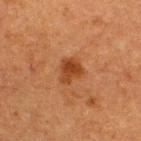The lesion was tiled from a total-body skin photograph and was not biopsied. A 15 mm crop from a total-body photograph taken for skin-cancer surveillance. Located on the upper back. The lesion's longest dimension is about 3 mm. This is a cross-polarized tile. A male patient about 50 years old.